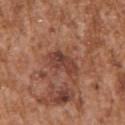Findings:
• workup — total-body-photography surveillance lesion; no biopsy
• location — the left upper arm
• illumination — white-light
• diameter — ≈4.5 mm
• acquisition — total-body-photography crop, ~15 mm field of view
• subject — male, aged 43–47